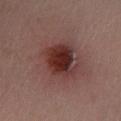biopsy status — imaged on a skin check; not biopsied | site — the left lower leg | size — ≈4.5 mm | TBP lesion metrics — an area of roughly 13 mm² and an eccentricity of roughly 0.6; a lesion–skin lightness drop of about 13; a nevus-likeness score of about 100/100 | acquisition — total-body-photography crop, ~15 mm field of view | subject — female, about 30 years old.